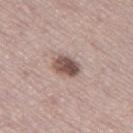biopsy status: no biopsy performed (imaged during a skin exam); anatomic site: the left thigh; subject: female, approximately 50 years of age; tile lighting: white-light; imaging modality: ~15 mm tile from a whole-body skin photo.